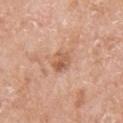{"biopsy_status": "not biopsied; imaged during a skin examination", "automated_metrics": {"area_mm2_approx": 5.0, "eccentricity": 0.65, "cielab_L": 59, "cielab_a": 23, "cielab_b": 33, "vs_skin_darker_L": 10.0, "vs_skin_contrast_norm": 6.5, "nevus_likeness_0_100": 0, "lesion_detection_confidence_0_100": 100}, "image": {"source": "total-body photography crop", "field_of_view_mm": 15}, "patient": {"sex": "female", "age_approx": "85+"}, "lighting": "white-light", "site": "right upper arm", "lesion_size": {"long_diameter_mm_approx": 3.0}}